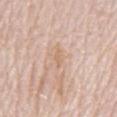No biopsy was performed on this lesion — it was imaged during a full skin examination and was not determined to be concerning. The recorded lesion diameter is about 2.5 mm. A male patient, roughly 80 years of age. A roughly 15 mm field-of-view crop from a total-body skin photograph. An algorithmic analysis of the crop reported an outline eccentricity of about 0.9 (0 = round, 1 = elongated). The analysis additionally found border irregularity of about 3 on a 0–10 scale, internal color variation of about 0 on a 0–10 scale, and peripheral color asymmetry of about 0. The analysis additionally found a lesion-detection confidence of about 90/100. The lesion is located on the chest.